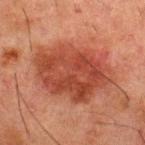<lesion>
  <biopsy_status>not biopsied; imaged during a skin examination</biopsy_status>
  <automated_metrics>
    <border_irregularity_0_10>2.5</border_irregularity_0_10>
    <peripheral_color_asymmetry>1.5</peripheral_color_asymmetry>
    <nevus_likeness_0_100>75</nevus_likeness_0_100>
    <lesion_detection_confidence_0_100>100</lesion_detection_confidence_0_100>
  </automated_metrics>
  <lesion_size>
    <long_diameter_mm_approx>8.5</long_diameter_mm_approx>
  </lesion_size>
  <image>
    <source>total-body photography crop</source>
    <field_of_view_mm>15</field_of_view_mm>
  </image>
  <patient>
    <sex>male</sex>
    <age_approx>50</age_approx>
  </patient>
  <site>upper back</site>
</lesion>